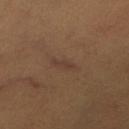<record>
<biopsy_status>not biopsied; imaged during a skin examination</biopsy_status>
</record>